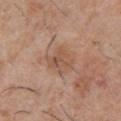<lesion>
<biopsy_status>not biopsied; imaged during a skin examination</biopsy_status>
<automated_metrics>
  <area_mm2_approx>7.5</area_mm2_approx>
  <eccentricity>0.35</eccentricity>
  <shape_asymmetry>0.25</shape_asymmetry>
  <cielab_L>53</cielab_L>
  <cielab_a>20</cielab_a>
  <cielab_b>30</cielab_b>
  <vs_skin_darker_L>8.0</vs_skin_darker_L>
  <vs_skin_contrast_norm>6.0</vs_skin_contrast_norm>
  <nevus_likeness_0_100>0</nevus_likeness_0_100>
  <lesion_detection_confidence_0_100>100</lesion_detection_confidence_0_100>
</automated_metrics>
<patient>
  <sex>male</sex>
  <age_approx>30</age_approx>
</patient>
<lighting>white-light</lighting>
<site>chest</site>
<image>
  <source>total-body photography crop</source>
  <field_of_view_mm>15</field_of_view_mm>
</image>
<lesion_size>
  <long_diameter_mm_approx>3.0</long_diameter_mm_approx>
</lesion_size>
</lesion>